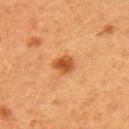Assessment: Captured during whole-body skin photography for melanoma surveillance; the lesion was not biopsied.